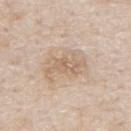Case summary:
– notes — catalogued during a skin exam; not biopsied
– image-analysis metrics — an area of roughly 11 mm² and an outline eccentricity of about 0.8 (0 = round, 1 = elongated); a lesion color around L≈65 a*≈14 b*≈30 in CIELAB and roughly 8 lightness units darker than nearby skin; a border-irregularity index near 6.5/10, internal color variation of about 4 on a 0–10 scale, and radial color variation of about 1.5; lesion-presence confidence of about 100/100
– acquisition — 15 mm crop, total-body photography
– illumination — white-light
– body site — the mid back
– patient — male, aged 63–67